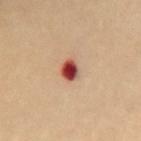{
  "biopsy_status": "not biopsied; imaged during a skin examination",
  "image": {
    "source": "total-body photography crop",
    "field_of_view_mm": 15
  },
  "site": "back",
  "patient": {
    "sex": "female",
    "age_approx": 60
  },
  "lighting": "cross-polarized",
  "automated_metrics": {
    "cielab_L": 48,
    "cielab_a": 32,
    "cielab_b": 29,
    "vs_skin_darker_L": 21.0,
    "vs_skin_contrast_norm": 14.0
  },
  "lesion_size": {
    "long_diameter_mm_approx": 2.5
  }
}